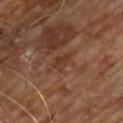biopsy_status: not biopsied; imaged during a skin examination
automated_metrics:
  cielab_L: 33
  cielab_a: 20
  cielab_b: 28
  vs_skin_darker_L: 5.0
lesion_size:
  long_diameter_mm_approx: 2.5
lighting: cross-polarized
site: chest
image:
  source: total-body photography crop
  field_of_view_mm: 15
patient:
  sex: male
  age_approx: 60